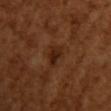Assessment:
The lesion was photographed on a routine skin check and not biopsied; there is no pathology result.
Image and clinical context:
The lesion is on the right upper arm. About 3.5 mm across. Imaged with cross-polarized lighting. A 15 mm crop from a total-body photograph taken for skin-cancer surveillance. A female patient, aged 53 to 57.